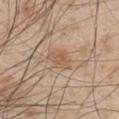biopsy_status: not biopsied; imaged during a skin examination
image:
  source: total-body photography crop
  field_of_view_mm: 15
automated_metrics:
  area_mm2_approx: 5.0
  eccentricity: 0.75
  shape_asymmetry: 0.3
  nevus_likeness_0_100: 25
  lesion_detection_confidence_0_100: 100
site: left upper arm
patient:
  sex: male
  age_approx: 50
lesion_size:
  long_diameter_mm_approx: 3.0
lighting: white-light